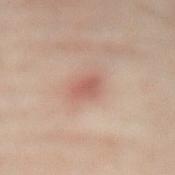biopsy status = no biopsy performed (imaged during a skin exam) | image = ~15 mm tile from a whole-body skin photo | patient = female, roughly 40 years of age | location = the abdomen.